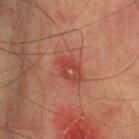Acquisition and patient details:
Approximately 3.5 mm at its widest. A roughly 15 mm field-of-view crop from a total-body skin photograph. Imaged with cross-polarized lighting. The lesion is on the left upper arm. The subject is a male in their mid-70s.